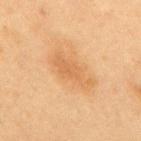  site: mid back
  image:
    source: total-body photography crop
    field_of_view_mm: 15
  patient:
    sex: female
    age_approx: 50
  lighting: cross-polarized
  automated_metrics:
    peripheral_color_asymmetry: 0.5
    nevus_likeness_0_100: 40